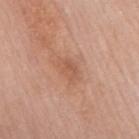The lesion was tiled from a total-body skin photograph and was not biopsied. A 15 mm close-up extracted from a 3D total-body photography capture. The subject is a male about 80 years old. The tile uses white-light illumination. On the mid back. The recorded lesion diameter is about 3 mm.